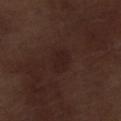biopsy status: imaged on a skin check; not biopsied | image source: ~15 mm crop, total-body skin-cancer survey | subject: male, in their 70s | tile lighting: white-light | TBP lesion metrics: a footprint of about 6 mm², a shape eccentricity near 0.7, and a symmetry-axis asymmetry near 0.25; a lesion color around L≈20 a*≈16 b*≈18 in CIELAB, roughly 4 lightness units darker than nearby skin, and a lesion-to-skin contrast of about 5.5 (normalized; higher = more distinct); a lesion-detection confidence of about 100/100 | anatomic site: the right lower leg.